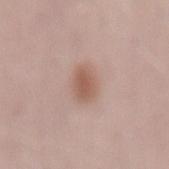Case summary:
– image: ~15 mm tile from a whole-body skin photo
– location: the lower back
– size: about 3 mm
– patient: female, aged 58–62
– image-analysis metrics: an area of roughly 5.5 mm², an outline eccentricity of about 0.75 (0 = round, 1 = elongated), and two-axis asymmetry of about 0.15; internal color variation of about 2 on a 0–10 scale and a peripheral color-asymmetry measure near 0.5; an automated nevus-likeness rating near 95 out of 100
– illumination: white-light illumination